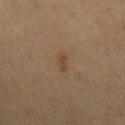biopsy status: no biopsy performed (imaged during a skin exam)
image source: 15 mm crop, total-body photography
site: the mid back
diameter: ≈2.5 mm
patient: male, aged around 70
illumination: cross-polarized illumination
automated metrics: a lesion color around L≈35 a*≈13 b*≈26 in CIELAB, roughly 5 lightness units darker than nearby skin, and a lesion-to-skin contrast of about 6 (normalized; higher = more distinct); an automated nevus-likeness rating near 20 out of 100